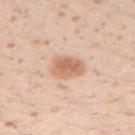Q: Was this lesion biopsied?
A: catalogued during a skin exam; not biopsied
Q: What kind of image is this?
A: ~15 mm tile from a whole-body skin photo
Q: What lighting was used for the tile?
A: white-light illumination
Q: Lesion location?
A: the arm
Q: How large is the lesion?
A: about 3.5 mm
Q: Patient demographics?
A: female, in their 20s